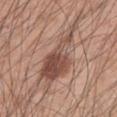Notes:
- notes · imaged on a skin check; not biopsied
- anatomic site · the left upper arm
- size · ~9 mm (longest diameter)
- acquisition · ~15 mm tile from a whole-body skin photo
- patient · male, aged 43–47